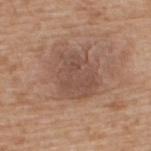The lesion's longest dimension is about 5.5 mm.
The lesion-visualizer software estimated an average lesion color of about L≈50 a*≈18 b*≈26 (CIELAB) and a lesion-to-skin contrast of about 5.5 (normalized; higher = more distinct). The software also gave a border-irregularity index near 2/10 and a within-lesion color-variation index near 3/10. The analysis additionally found a classifier nevus-likeness of about 0/100 and a detector confidence of about 100 out of 100 that the crop contains a lesion.
A roughly 15 mm field-of-view crop from a total-body skin photograph.
A male subject roughly 65 years of age.
From the upper back.
Imaged with white-light lighting.